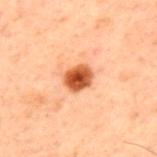Q: Was this lesion biopsied?
A: no biopsy performed (imaged during a skin exam)
Q: What are the patient's age and sex?
A: male, aged around 60
Q: How was this image acquired?
A: ~15 mm crop, total-body skin-cancer survey
Q: Automated lesion metrics?
A: a shape eccentricity near 0.55; a lesion color around L≈44 a*≈26 b*≈35 in CIELAB; a nevus-likeness score of about 100/100 and a lesion-detection confidence of about 100/100
Q: What lighting was used for the tile?
A: cross-polarized
Q: What is the anatomic site?
A: the upper back
Q: How large is the lesion?
A: ~3 mm (longest diameter)